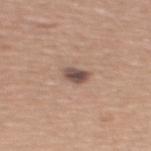The lesion was tiled from a total-body skin photograph and was not biopsied. A 15 mm close-up extracted from a 3D total-body photography capture. Longest diameter approximately 2.5 mm. Imaged with white-light lighting. Located on the mid back. A female subject aged 53–57.